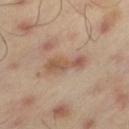biopsy status: imaged on a skin check; not biopsied | body site: the left thigh | image: 15 mm crop, total-body photography | subject: male, aged 43–47 | tile lighting: cross-polarized | image-analysis metrics: an area of roughly 8 mm², an eccentricity of roughly 0.95, and a shape-asymmetry score of about 0.3 (0 = symmetric); a mean CIELAB color near L≈53 a*≈18 b*≈28 and about 9 CIELAB-L* units darker than the surrounding skin.